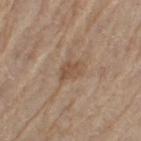Captured during whole-body skin photography for melanoma surveillance; the lesion was not biopsied. The lesion's longest dimension is about 3 mm. An algorithmic analysis of the crop reported an average lesion color of about L≈51 a*≈16 b*≈30 (CIELAB), a lesion–skin lightness drop of about 8, and a lesion-to-skin contrast of about 6 (normalized; higher = more distinct). It also reported a within-lesion color-variation index near 2/10 and peripheral color asymmetry of about 1. The lesion is on the leg. Captured under white-light illumination. A close-up tile cropped from a whole-body skin photograph, about 15 mm across. A male subject roughly 70 years of age.